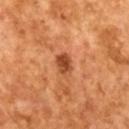<tbp_lesion>
  <biopsy_status>not biopsied; imaged during a skin examination</biopsy_status>
</tbp_lesion>